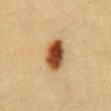  biopsy_status: not biopsied; imaged during a skin examination
  lesion_size:
    long_diameter_mm_approx: 4.5
  image:
    source: total-body photography crop
    field_of_view_mm: 15
  patient:
    sex: female
    age_approx: 30
  lighting: cross-polarized
  automated_metrics:
    border_irregularity_0_10: 1.0
    color_variation_0_10: 8.5
    peripheral_color_asymmetry: 2.5
    nevus_likeness_0_100: 100
    lesion_detection_confidence_0_100: 100
  site: mid back